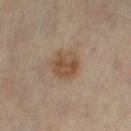Findings:
- notes: no biopsy performed (imaged during a skin exam)
- body site: the left thigh
- image: ~15 mm crop, total-body skin-cancer survey
- patient: female, aged around 50